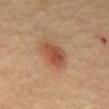Findings:
* follow-up · no biopsy performed (imaged during a skin exam)
* lesion size · about 4 mm
* subject · female, roughly 65 years of age
* location · the mid back
* illumination · cross-polarized
* image · 15 mm crop, total-body photography